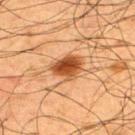Q: Was this lesion biopsied?
A: no biopsy performed (imaged during a skin exam)
Q: Automated lesion metrics?
A: a footprint of about 8.5 mm² and a shape-asymmetry score of about 0.25 (0 = symmetric); a mean CIELAB color near L≈40 a*≈23 b*≈34 and roughly 13 lightness units darker than nearby skin; a classifier nevus-likeness of about 100/100
Q: How large is the lesion?
A: ~4 mm (longest diameter)
Q: What kind of image is this?
A: total-body-photography crop, ~15 mm field of view
Q: Lesion location?
A: the back
Q: Patient demographics?
A: male, aged around 60